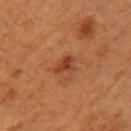Findings:
- biopsy status — imaged on a skin check; not biopsied
- anatomic site — the left upper arm
- lesion diameter — about 2.5 mm
- patient — female, roughly 50 years of age
- imaging modality — 15 mm crop, total-body photography
- tile lighting — cross-polarized